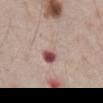Background: The lesion is located on the abdomen. A male subject, in their mid- to late 60s. Longest diameter approximately 5.5 mm. Cropped from a total-body skin-imaging series; the visible field is about 15 mm. This is a white-light tile.